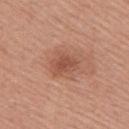A female patient, in their mid-50s. Cropped from a total-body skin-imaging series; the visible field is about 15 mm. This is a white-light tile. Located on the right upper arm. Automated image analysis of the tile measured a mean CIELAB color near L≈52 a*≈24 b*≈31. The analysis additionally found a nevus-likeness score of about 10/100 and a lesion-detection confidence of about 100/100.